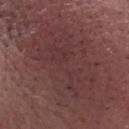On the head or neck.
A male patient aged around 55.
Automated image analysis of the tile measured a shape eccentricity near 0.85 and a symmetry-axis asymmetry near 0.3. And it measured an average lesion color of about L≈37 a*≈21 b*≈17 (CIELAB), about 7 CIELAB-L* units darker than the surrounding skin, and a normalized border contrast of about 6.
Measured at roughly 18.5 mm in maximum diameter.
A 15 mm close-up tile from a total-body photography series done for melanoma screening.
The tile uses white-light illumination.